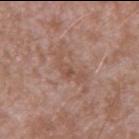Recorded during total-body skin imaging; not selected for excision or biopsy. This image is a 15 mm lesion crop taken from a total-body photograph. A male subject aged 43 to 47. The lesion is on the right upper arm.